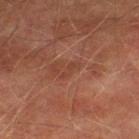Case summary:
• notes — no biopsy performed (imaged during a skin exam)
• image-analysis metrics — a mean CIELAB color near L≈33 a*≈21 b*≈25, a lesion–skin lightness drop of about 5, and a normalized lesion–skin contrast near 4.5; a classifier nevus-likeness of about 0/100 and lesion-presence confidence of about 100/100
• tile lighting — cross-polarized
• image — ~15 mm tile from a whole-body skin photo
• anatomic site — the left thigh
• subject — male, aged 73 to 77
• lesion size — ~3 mm (longest diameter)